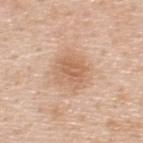From the upper back. A male subject approximately 50 years of age. About 4.5 mm across. A region of skin cropped from a whole-body photographic capture, roughly 15 mm wide.